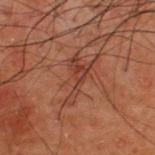Q: How was this image acquired?
A: 15 mm crop, total-body photography
Q: Lesion location?
A: the upper back
Q: What are the patient's age and sex?
A: male, in their 50s